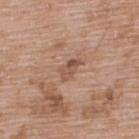workup — catalogued during a skin exam; not biopsied
illumination — white-light
image source — ~15 mm tile from a whole-body skin photo
anatomic site — the upper back
subject — male, aged 48 to 52
size — about 3 mm